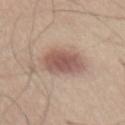notes=catalogued during a skin exam; not biopsied
location=the abdomen
size=about 5 mm
illumination=white-light illumination
acquisition=total-body-photography crop, ~15 mm field of view
patient=male, aged 53–57